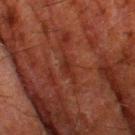The lesion is on the left thigh.
This image is a 15 mm lesion crop taken from a total-body photograph.
A male subject aged 78–82.